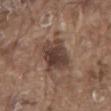The lesion was photographed on a routine skin check and not biopsied; there is no pathology result. Measured at roughly 5 mm in maximum diameter. A male subject, about 80 years old. The lesion is located on the mid back. The lesion-visualizer software estimated a footprint of about 14 mm², an outline eccentricity of about 0.7 (0 = round, 1 = elongated), and two-axis asymmetry of about 0.2. This is a white-light tile. A close-up tile cropped from a whole-body skin photograph, about 15 mm across.